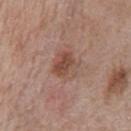Q: Was this lesion biopsied?
A: catalogued during a skin exam; not biopsied
Q: What lighting was used for the tile?
A: white-light illumination
Q: What did automated image analysis measure?
A: an average lesion color of about L≈48 a*≈20 b*≈27 (CIELAB), roughly 9 lightness units darker than nearby skin, and a normalized border contrast of about 7.5; internal color variation of about 5 on a 0–10 scale; an automated nevus-likeness rating near 65 out of 100 and a detector confidence of about 100 out of 100 that the crop contains a lesion
Q: Patient demographics?
A: male, aged approximately 70
Q: Lesion location?
A: the chest
Q: What kind of image is this?
A: total-body-photography crop, ~15 mm field of view
Q: What is the lesion's diameter?
A: about 3.5 mm